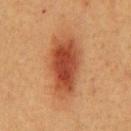<tbp_lesion>
  <biopsy_status>not biopsied; imaged during a skin examination</biopsy_status>
  <lighting>cross-polarized</lighting>
  <automated_metrics>
    <cielab_L>45</cielab_L>
    <cielab_a>27</cielab_a>
    <cielab_b>35</cielab_b>
    <vs_skin_darker_L>13.0</vs_skin_darker_L>
    <vs_skin_contrast_norm>10.0</vs_skin_contrast_norm>
    <nevus_likeness_0_100>100</nevus_likeness_0_100>
    <lesion_detection_confidence_0_100>100</lesion_detection_confidence_0_100>
  </automated_metrics>
  <image>
    <source>total-body photography crop</source>
    <field_of_view_mm>15</field_of_view_mm>
  </image>
  <site>front of the torso</site>
  <patient>
    <sex>male</sex>
    <age_approx>60</age_approx>
  </patient>
</tbp_lesion>